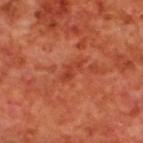TBP lesion metrics — a footprint of about 3 mm² and a shape-asymmetry score of about 0.5 (0 = symmetric); an average lesion color of about L≈41 a*≈34 b*≈36 (CIELAB), roughly 7 lightness units darker than nearby skin, and a normalized lesion–skin contrast near 6; an automated nevus-likeness rating near 0 out of 100 and lesion-presence confidence of about 100/100 | illumination — cross-polarized illumination | image — 15 mm crop, total-body photography | body site — the upper back | lesion size — ~3 mm (longest diameter) | subject — male, aged around 70.